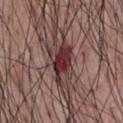Case summary:
– notes — catalogued during a skin exam; not biopsied
– imaging modality — total-body-photography crop, ~15 mm field of view
– anatomic site — the chest
– lesion diameter — ≈5 mm
– illumination — white-light illumination
– patient — male, in their mid- to late 50s